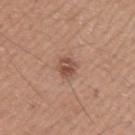Q: How large is the lesion?
A: about 2.5 mm
Q: Where on the body is the lesion?
A: the left upper arm
Q: What did automated image analysis measure?
A: an automated nevus-likeness rating near 65 out of 100 and a detector confidence of about 100 out of 100 that the crop contains a lesion
Q: What is the imaging modality?
A: ~15 mm crop, total-body skin-cancer survey
Q: Patient demographics?
A: male, aged 18–22
Q: How was the tile lit?
A: white-light illumination